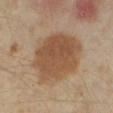automated metrics: an area of roughly 31 mm², an eccentricity of roughly 0.55, and two-axis asymmetry of about 0.15; a lesion color around L≈48 a*≈17 b*≈31 in CIELAB, about 10 CIELAB-L* units darker than the surrounding skin, and a normalized lesion–skin contrast near 8; a border-irregularity rating of about 1.5/10, internal color variation of about 3 on a 0–10 scale, and radial color variation of about 1; a classifier nevus-likeness of about 90/100
image source: ~15 mm crop, total-body skin-cancer survey
lighting: cross-polarized illumination
subject: female, in their mid-30s
body site: the right lower leg